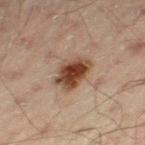notes=imaged on a skin check; not biopsied | location=the left thigh | patient=male, aged 43–47 | acquisition=~15 mm crop, total-body skin-cancer survey.